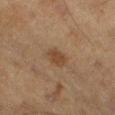This lesion was catalogued during total-body skin photography and was not selected for biopsy. The lesion is located on the left thigh. Captured under cross-polarized illumination. Cropped from a whole-body photographic skin survey; the tile spans about 15 mm. Automated tile analysis of the lesion measured a within-lesion color-variation index near 1.5/10. The software also gave a nevus-likeness score of about 55/100 and a detector confidence of about 100 out of 100 that the crop contains a lesion. The lesion's longest dimension is about 2.5 mm. A male patient aged around 75.